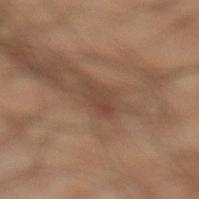workup = no biopsy performed (imaged during a skin exam) | image = 15 mm crop, total-body photography | lesion size = ~3.5 mm (longest diameter) | anatomic site = the left lower leg | subject = male, about 50 years old | lighting = cross-polarized.